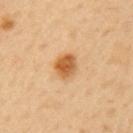workup = no biopsy performed (imaged during a skin exam); lighting = cross-polarized illumination; diameter = ~3 mm (longest diameter); subject = female, aged 48–52; automated metrics = an automated nevus-likeness rating near 100 out of 100 and lesion-presence confidence of about 100/100; body site = the arm; imaging modality = total-body-photography crop, ~15 mm field of view.